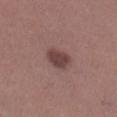<record>
<biopsy_status>not biopsied; imaged during a skin examination</biopsy_status>
<site>right lower leg</site>
<automated_metrics>
  <border_irregularity_0_10>2.0</border_irregularity_0_10>
  <color_variation_0_10>2.0</color_variation_0_10>
  <peripheral_color_asymmetry>0.5</peripheral_color_asymmetry>
  <nevus_likeness_0_100>70</nevus_likeness_0_100>
</automated_metrics>
<lighting>white-light</lighting>
<patient>
  <sex>female</sex>
  <age_approx>20</age_approx>
</patient>
<image>
  <source>total-body photography crop</source>
  <field_of_view_mm>15</field_of_view_mm>
</image>
<lesion_size>
  <long_diameter_mm_approx>3.5</long_diameter_mm_approx>
</lesion_size>
</record>